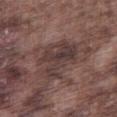biopsy status: no biopsy performed (imaged during a skin exam).